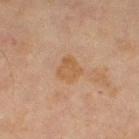Background:
A female patient aged 68–72. A region of skin cropped from a whole-body photographic capture, roughly 15 mm wide. The lesion is located on the right thigh.